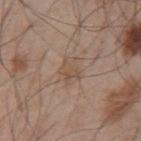This lesion was catalogued during total-body skin photography and was not selected for biopsy.
A male subject, roughly 55 years of age.
Cropped from a whole-body photographic skin survey; the tile spans about 15 mm.
Located on the back.
The tile uses white-light illumination.
About 3.5 mm across.